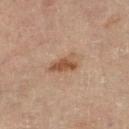Acquisition and patient details: This image is a 15 mm lesion crop taken from a total-body photograph. Located on the left leg. Automated tile analysis of the lesion measured a footprint of about 5 mm², an eccentricity of roughly 0.85, and a shape-asymmetry score of about 0.4 (0 = symmetric). It also reported a classifier nevus-likeness of about 60/100 and a lesion-detection confidence of about 100/100. Longest diameter approximately 3.5 mm. Captured under cross-polarized illumination. A female subject, about 80 years old.